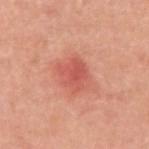The lesion was tiled from a total-body skin photograph and was not biopsied. Cropped from a whole-body photographic skin survey; the tile spans about 15 mm. Longest diameter approximately 3.5 mm. A male patient, in their 50s. Automated tile analysis of the lesion measured a within-lesion color-variation index near 3.5/10. The tile uses white-light illumination. On the arm.